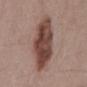Part of a total-body skin-imaging series; this lesion was reviewed on a skin check and was not flagged for biopsy.